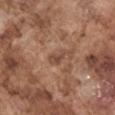Q: Is there a histopathology result?
A: total-body-photography surveillance lesion; no biopsy
Q: What kind of image is this?
A: 15 mm crop, total-body photography
Q: What did automated image analysis measure?
A: a lesion area of about 3 mm², an outline eccentricity of about 0.8 (0 = round, 1 = elongated), and a symmetry-axis asymmetry near 0.4; a mean CIELAB color near L≈47 a*≈20 b*≈28
Q: Illumination type?
A: white-light
Q: What is the lesion's diameter?
A: ~2.5 mm (longest diameter)
Q: What are the patient's age and sex?
A: male, aged 73–77
Q: Where on the body is the lesion?
A: the arm